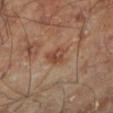{"biopsy_status": "not biopsied; imaged during a skin examination", "lesion_size": {"long_diameter_mm_approx": 2.5}, "lighting": "cross-polarized", "patient": {"sex": "male", "age_approx": 70}, "site": "left lower leg", "automated_metrics": {"area_mm2_approx": 4.5, "eccentricity": 0.6, "shape_asymmetry": 0.35, "cielab_L": 44, "cielab_a": 21, "cielab_b": 29, "vs_skin_darker_L": 9.0, "vs_skin_contrast_norm": 7.5, "border_irregularity_0_10": 3.0, "color_variation_0_10": 4.0, "peripheral_color_asymmetry": 1.5, "lesion_detection_confidence_0_100": 100}, "image": {"source": "total-body photography crop", "field_of_view_mm": 15}}